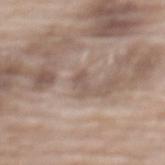notes: no biopsy performed (imaged during a skin exam) | acquisition: ~15 mm tile from a whole-body skin photo | body site: the back | image-analysis metrics: a lesion area of about 2.5 mm², an outline eccentricity of about 0.9 (0 = round, 1 = elongated), and a symmetry-axis asymmetry near 0.45; an average lesion color of about L≈54 a*≈15 b*≈23 (CIELAB), roughly 7 lightness units darker than nearby skin, and a normalized lesion–skin contrast near 5.5 | subject: female, aged approximately 75 | lesion size: ~2.5 mm (longest diameter).